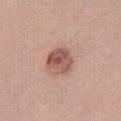follow-up=imaged on a skin check; not biopsied
image=~15 mm tile from a whole-body skin photo
lesion diameter=≈3.5 mm
anatomic site=the right thigh
patient=female, aged 33 to 37
lighting=white-light
automated lesion analysis=a mean CIELAB color near L≈54 a*≈23 b*≈25, roughly 13 lightness units darker than nearby skin, and a normalized border contrast of about 8.5; a peripheral color-asymmetry measure near 2